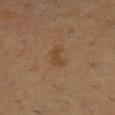This lesion was catalogued during total-body skin photography and was not selected for biopsy.